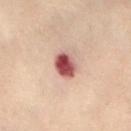| key | value |
|---|---|
| notes | imaged on a skin check; not biopsied |
| automated lesion analysis | a footprint of about 6.5 mm² and a symmetry-axis asymmetry near 0.15; a lesion color around L≈46 a*≈25 b*≈21 in CIELAB, about 18 CIELAB-L* units darker than the surrounding skin, and a lesion-to-skin contrast of about 13 (normalized; higher = more distinct); an automated nevus-likeness rating near 0 out of 100 and a detector confidence of about 100 out of 100 that the crop contains a lesion |
| illumination | cross-polarized |
| patient | female, roughly 60 years of age |
| location | the abdomen |
| acquisition | ~15 mm crop, total-body skin-cancer survey |
| diameter | about 3 mm |